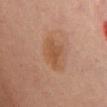Q: Was this lesion biopsied?
A: no biopsy performed (imaged during a skin exam)
Q: What is the lesion's diameter?
A: ≈3.5 mm
Q: Automated lesion metrics?
A: an area of roughly 6.5 mm² and a shape eccentricity near 0.7; a lesion color around L≈47 a*≈20 b*≈32 in CIELAB, roughly 7 lightness units darker than nearby skin, and a lesion-to-skin contrast of about 6.5 (normalized; higher = more distinct); border irregularity of about 4 on a 0–10 scale, a color-variation rating of about 3/10, and a peripheral color-asymmetry measure near 1
Q: Lesion location?
A: the chest
Q: Illumination type?
A: cross-polarized illumination
Q: Who is the patient?
A: female, aged 58 to 62
Q: What is the imaging modality?
A: ~15 mm tile from a whole-body skin photo